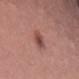biopsy_status: not biopsied; imaged during a skin examination
patient:
  sex: female
  age_approx: 35
site: left thigh
lesion_size:
  long_diameter_mm_approx: 3.5
lighting: white-light
automated_metrics:
  eccentricity: 0.85
  shape_asymmetry: 0.25
  border_irregularity_0_10: 3.0
  peripheral_color_asymmetry: 1.5
image:
  source: total-body photography crop
  field_of_view_mm: 15